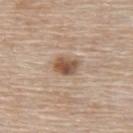No biopsy was performed on this lesion — it was imaged during a full skin examination and was not determined to be concerning.
A 15 mm close-up tile from a total-body photography series done for melanoma screening.
A female subject, aged 63 to 67.
From the back.